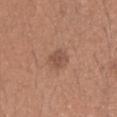Impression:
Imaged during a routine full-body skin examination; the lesion was not biopsied and no histopathology is available.
Acquisition and patient details:
A female patient aged 38 to 42. An algorithmic analysis of the crop reported a nevus-likeness score of about 55/100 and lesion-presence confidence of about 100/100. Approximately 2.5 mm at its widest. The tile uses white-light illumination. A lesion tile, about 15 mm wide, cut from a 3D total-body photograph. The lesion is located on the head or neck.